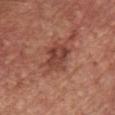notes = imaged on a skin check; not biopsied | body site = the chest | lighting = white-light | imaging modality = ~15 mm tile from a whole-body skin photo | subject = male, in their mid- to late 60s | automated lesion analysis = a lesion area of about 10 mm² and a shape eccentricity near 0.65; a lesion–skin lightness drop of about 10 and a normalized border contrast of about 7.5; an automated nevus-likeness rating near 0 out of 100 and a detector confidence of about 100 out of 100 that the crop contains a lesion.